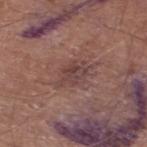A roughly 15 mm field-of-view crop from a total-body skin photograph. This is a white-light tile. The recorded lesion diameter is about 3.5 mm. The lesion is located on the left lower leg. A male subject aged approximately 70. Automated tile analysis of the lesion measured a shape-asymmetry score of about 0.3 (0 = symmetric). The software also gave a classifier nevus-likeness of about 0/100.